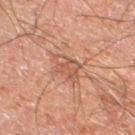{
  "biopsy_status": "not biopsied; imaged during a skin examination",
  "automated_metrics": {
    "area_mm2_approx": 7.5,
    "eccentricity": 0.7,
    "shape_asymmetry": 0.35,
    "cielab_L": 50,
    "cielab_a": 22,
    "cielab_b": 29,
    "vs_skin_contrast_norm": 5.0
  },
  "image": {
    "source": "total-body photography crop",
    "field_of_view_mm": 15
  },
  "lesion_size": {
    "long_diameter_mm_approx": 3.5
  },
  "patient": {
    "sex": "male",
    "age_approx": 60
  },
  "site": "right thigh"
}